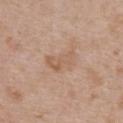| field | value |
|---|---|
| automated metrics | an area of roughly 7 mm² and an outline eccentricity of about 0.8 (0 = round, 1 = elongated); a lesion color around L≈59 a*≈18 b*≈31 in CIELAB, about 6 CIELAB-L* units darker than the surrounding skin, and a lesion-to-skin contrast of about 5 (normalized; higher = more distinct); a border-irregularity index near 4/10, a color-variation rating of about 3.5/10, and peripheral color asymmetry of about 1; an automated nevus-likeness rating near 0 out of 100 and a detector confidence of about 100 out of 100 that the crop contains a lesion |
| subject | female, aged 28 to 32 |
| image | ~15 mm crop, total-body skin-cancer survey |
| location | the upper back |